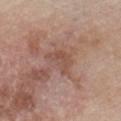| key | value |
|---|---|
| follow-up | no biopsy performed (imaged during a skin exam) |
| TBP lesion metrics | a lesion color around L≈51 a*≈20 b*≈27 in CIELAB, about 6 CIELAB-L* units darker than the surrounding skin, and a normalized lesion–skin contrast near 5 |
| site | the chest |
| imaging modality | total-body-photography crop, ~15 mm field of view |
| lighting | white-light |
| patient | male, aged approximately 85 or older |
| size | ~4 mm (longest diameter) |